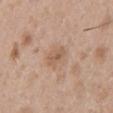Assessment:
The lesion was photographed on a routine skin check and not biopsied; there is no pathology result.
Acquisition and patient details:
The tile uses white-light illumination. Approximately 3 mm at its widest. From the mid back. The total-body-photography lesion software estimated an average lesion color of about L≈57 a*≈18 b*≈30 (CIELAB), about 8 CIELAB-L* units darker than the surrounding skin, and a normalized border contrast of about 6. The subject is a male aged around 50. A region of skin cropped from a whole-body photographic capture, roughly 15 mm wide.